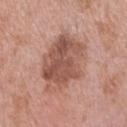<tbp_lesion>
<biopsy_status>not biopsied; imaged during a skin examination</biopsy_status>
<patient>
  <sex>male</sex>
  <age_approx>50</age_approx>
</patient>
<image>
  <source>total-body photography crop</source>
  <field_of_view_mm>15</field_of_view_mm>
</image>
<lesion_size>
  <long_diameter_mm_approx>7.5</long_diameter_mm_approx>
</lesion_size>
<site>chest</site>
</tbp_lesion>